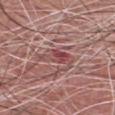Q: Is there a histopathology result?
A: imaged on a skin check; not biopsied